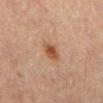subject = male, aged approximately 60
body site = the abdomen
image source = total-body-photography crop, ~15 mm field of view